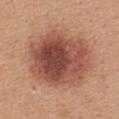workup: total-body-photography surveillance lesion; no biopsy
diameter: about 9 mm
tile lighting: white-light
automated metrics: an area of roughly 45 mm², an outline eccentricity of about 0.65 (0 = round, 1 = elongated), and two-axis asymmetry of about 0.1; a classifier nevus-likeness of about 90/100 and a detector confidence of about 100 out of 100 that the crop contains a lesion
subject: female, about 45 years old
image source: 15 mm crop, total-body photography
anatomic site: the back